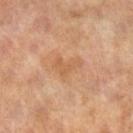| field | value |
|---|---|
| lighting | cross-polarized illumination |
| lesion size | ≈4 mm |
| patient | female, about 60 years old |
| image | total-body-photography crop, ~15 mm field of view |
| body site | the right lower leg |
| automated lesion analysis | a within-lesion color-variation index near 2/10 and peripheral color asymmetry of about 0.5; lesion-presence confidence of about 100/100 |